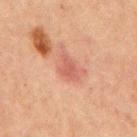Findings:
– notes — total-body-photography surveillance lesion; no biopsy
– lighting — cross-polarized
– diameter — about 3 mm
– subject — male, aged 63–67
– image — ~15 mm tile from a whole-body skin photo
– location — the chest